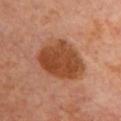  biopsy_status: not biopsied; imaged during a skin examination